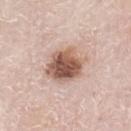{
  "biopsy_status": "not biopsied; imaged during a skin examination",
  "lesion_size": {
    "long_diameter_mm_approx": 5.0
  },
  "automated_metrics": {
    "border_irregularity_0_10": 2.0,
    "color_variation_0_10": 7.5,
    "peripheral_color_asymmetry": 2.5,
    "nevus_likeness_0_100": 85,
    "lesion_detection_confidence_0_100": 100
  },
  "patient": {
    "sex": "male",
    "age_approx": 80
  },
  "site": "left lower leg",
  "lighting": "white-light",
  "image": {
    "source": "total-body photography crop",
    "field_of_view_mm": 15
  }
}